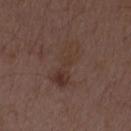{"biopsy_status": "not biopsied; imaged during a skin examination", "image": {"source": "total-body photography crop", "field_of_view_mm": 15}, "patient": {"sex": "male", "age_approx": 50}}